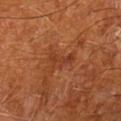- notes — imaged on a skin check; not biopsied
- location — the left lower leg
- tile lighting — cross-polarized illumination
- size — about 3.5 mm
- subject — male, approximately 65 years of age
- imaging modality — ~15 mm crop, total-body skin-cancer survey